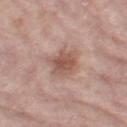follow-up: no biopsy performed (imaged during a skin exam) | lighting: white-light illumination | diameter: about 3.5 mm | patient: female, about 70 years old | automated lesion analysis: a footprint of about 8.5 mm², an eccentricity of roughly 0.5, and a shape-asymmetry score of about 0.25 (0 = symmetric); a lesion color around L≈54 a*≈21 b*≈26 in CIELAB and about 11 CIELAB-L* units darker than the surrounding skin; a within-lesion color-variation index near 3/10 and a peripheral color-asymmetry measure near 1; a classifier nevus-likeness of about 30/100 and a lesion-detection confidence of about 100/100 | site: the left thigh | image source: 15 mm crop, total-body photography.